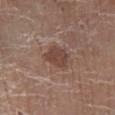{
  "biopsy_status": "not biopsied; imaged during a skin examination",
  "site": "leg",
  "lighting": "white-light",
  "patient": {
    "sex": "female",
    "age_approx": 80
  },
  "image": {
    "source": "total-body photography crop",
    "field_of_view_mm": 15
  }
}